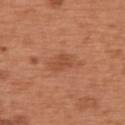Findings:
- notes · imaged on a skin check; not biopsied
- anatomic site · the back
- acquisition · 15 mm crop, total-body photography
- patient · male, aged 63–67
- lighting · white-light illumination